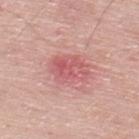{
  "biopsy_status": "not biopsied; imaged during a skin examination",
  "patient": {
    "sex": "male",
    "age_approx": 50
  },
  "image": {
    "source": "total-body photography crop",
    "field_of_view_mm": 15
  },
  "lighting": "white-light",
  "site": "upper back"
}